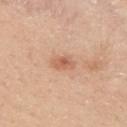{"biopsy_status": "not biopsied; imaged during a skin examination", "lesion_size": {"long_diameter_mm_approx": 3.0}, "site": "upper back", "automated_metrics": {"cielab_L": 61, "cielab_a": 23, "cielab_b": 32, "vs_skin_contrast_norm": 6.5}, "patient": {"sex": "male", "age_approx": 30}, "image": {"source": "total-body photography crop", "field_of_view_mm": 15}}